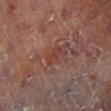biopsy status = total-body-photography surveillance lesion; no biopsy
site = the left leg
imaging modality = ~15 mm tile from a whole-body skin photo
patient = female, aged approximately 80